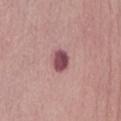Captured under white-light illumination.
The subject is a female about 85 years old or older.
Approximately 3 mm at its widest.
From the abdomen.
Cropped from a total-body skin-imaging series; the visible field is about 15 mm.